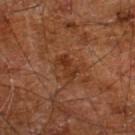{
  "biopsy_status": "not biopsied; imaged during a skin examination",
  "image": {
    "source": "total-body photography crop",
    "field_of_view_mm": 15
  },
  "patient": {
    "sex": "male",
    "age_approx": 60
  },
  "automated_metrics": {
    "area_mm2_approx": 4.5,
    "eccentricity": 0.85,
    "shape_asymmetry": 0.3
  },
  "lighting": "cross-polarized",
  "site": "right leg",
  "lesion_size": {
    "long_diameter_mm_approx": 3.0
  }
}